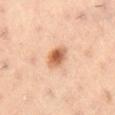The lesion was photographed on a routine skin check and not biopsied; there is no pathology result. On the right thigh. Approximately 2.5 mm at its widest. Imaged with cross-polarized lighting. The patient is a male about 55 years old. This image is a 15 mm lesion crop taken from a total-body photograph. The lesion-visualizer software estimated roughly 14 lightness units darker than nearby skin and a normalized border contrast of about 9. And it measured internal color variation of about 5.5 on a 0–10 scale and radial color variation of about 1.5. The software also gave a nevus-likeness score of about 100/100 and lesion-presence confidence of about 100/100.